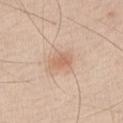Impression:
Captured during whole-body skin photography for melanoma surveillance; the lesion was not biopsied.
Image and clinical context:
The patient is a male aged around 45. An algorithmic analysis of the crop reported a lesion color around L≈64 a*≈20 b*≈32 in CIELAB, a lesion–skin lightness drop of about 9, and a normalized lesion–skin contrast near 6. The lesion is on the chest. Imaged with white-light lighting. Longest diameter approximately 3 mm. A roughly 15 mm field-of-view crop from a total-body skin photograph.